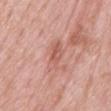Clinical summary:
The tile uses white-light illumination. On the mid back. A region of skin cropped from a whole-body photographic capture, roughly 15 mm wide. The lesion's longest dimension is about 5 mm. Automated image analysis of the tile measured a classifier nevus-likeness of about 0/100 and lesion-presence confidence of about 95/100. The patient is a female aged 58–62.